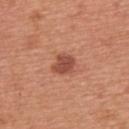{
  "biopsy_status": "not biopsied; imaged during a skin examination",
  "image": {
    "source": "total-body photography crop",
    "field_of_view_mm": 15
  },
  "patient": {
    "sex": "male",
    "age_approx": 65
  },
  "lesion_size": {
    "long_diameter_mm_approx": 2.5
  },
  "site": "upper back",
  "lighting": "white-light"
}